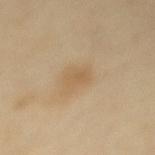biopsy_status: not biopsied; imaged during a skin examination
lighting: cross-polarized
automated_metrics:
  area_mm2_approx: 4.5
  vs_skin_darker_L: 7.0
  vs_skin_contrast_norm: 5.0
lesion_size:
  long_diameter_mm_approx: 2.5
site: mid back
image:
  source: total-body photography crop
  field_of_view_mm: 15
patient:
  sex: female
  age_approx: 40